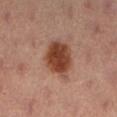Imaged during a routine full-body skin examination; the lesion was not biopsied and no histopathology is available. The patient is a female in their mid- to late 30s. This image is a 15 mm lesion crop taken from a total-body photograph. Automated image analysis of the tile measured an area of roughly 14 mm² and an outline eccentricity of about 0.6 (0 = round, 1 = elongated). Measured at roughly 5 mm in maximum diameter. From the left lower leg. Imaged with cross-polarized lighting.